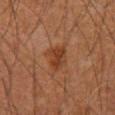Assessment: Recorded during total-body skin imaging; not selected for excision or biopsy. Acquisition and patient details: Automated image analysis of the tile measured an average lesion color of about L≈36 a*≈22 b*≈31 (CIELAB), about 7 CIELAB-L* units darker than the surrounding skin, and a normalized border contrast of about 7. The software also gave a border-irregularity rating of about 3/10, a color-variation rating of about 4/10, and a peripheral color-asymmetry measure near 1.5. It also reported a detector confidence of about 100 out of 100 that the crop contains a lesion. The recorded lesion diameter is about 3.5 mm. Imaged with cross-polarized lighting. The lesion is on the mid back. A male subject, aged around 60. A 15 mm close-up extracted from a 3D total-body photography capture.